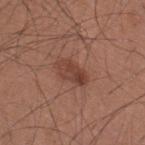Recorded during total-body skin imaging; not selected for excision or biopsy.
The patient is a male aged 33 to 37.
The recorded lesion diameter is about 4 mm.
The lesion is on the upper back.
A close-up tile cropped from a whole-body skin photograph, about 15 mm across.